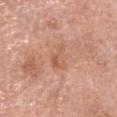biopsy status=total-body-photography surveillance lesion; no biopsy
site=the head or neck
subject=female, in their 60s
imaging modality=~15 mm tile from a whole-body skin photo